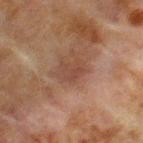Part of a total-body skin-imaging series; this lesion was reviewed on a skin check and was not flagged for biopsy. A 15 mm close-up tile from a total-body photography series done for melanoma screening. A male patient in their mid-60s. The lesion-visualizer software estimated a shape eccentricity near 0.65 and two-axis asymmetry of about 0.35. And it measured an automated nevus-likeness rating near 0 out of 100 and lesion-presence confidence of about 100/100. The tile uses cross-polarized illumination. From the chest. Measured at roughly 2.5 mm in maximum diameter.